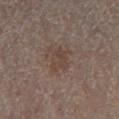lighting: cross-polarized | image: ~15 mm crop, total-body skin-cancer survey | subject: female, roughly 80 years of age | size: ~3.5 mm (longest diameter) | site: the leg.